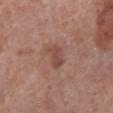biopsy status = no biopsy performed (imaged during a skin exam).